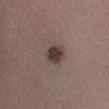Case summary:
* biopsy status: total-body-photography surveillance lesion; no biopsy
* illumination: white-light
* anatomic site: the leg
* TBP lesion metrics: an area of roughly 5 mm², an outline eccentricity of about 0.45 (0 = round, 1 = elongated), and a shape-asymmetry score of about 0.2 (0 = symmetric); a mean CIELAB color near L≈37 a*≈13 b*≈15, about 13 CIELAB-L* units darker than the surrounding skin, and a normalized lesion–skin contrast near 11; internal color variation of about 2.5 on a 0–10 scale and a peripheral color-asymmetry measure near 0.5
* image: total-body-photography crop, ~15 mm field of view
* subject: male, aged 38 to 42
* lesion size: ≈2.5 mm